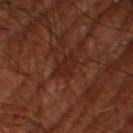This lesion was catalogued during total-body skin photography and was not selected for biopsy.
A region of skin cropped from a whole-body photographic capture, roughly 15 mm wide.
Longest diameter approximately 3.5 mm.
Imaged with cross-polarized lighting.
The patient is a male aged around 70.
Located on the leg.
Automated tile analysis of the lesion measured a color-variation rating of about 2.5/10 and peripheral color asymmetry of about 1.